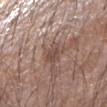Part of a total-body skin-imaging series; this lesion was reviewed on a skin check and was not flagged for biopsy.
From the right upper arm.
A male subject about 70 years old.
Imaged with white-light lighting.
A lesion tile, about 15 mm wide, cut from a 3D total-body photograph.